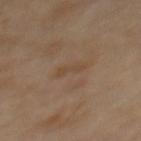Recorded during total-body skin imaging; not selected for excision or biopsy. A close-up tile cropped from a whole-body skin photograph, about 15 mm across. From the mid back.